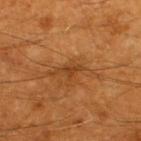  biopsy_status: not biopsied; imaged during a skin examination
  site: upper back
  patient:
    sex: male
    age_approx: 60
  lesion_size:
    long_diameter_mm_approx: 3.0
  lighting: cross-polarized
  automated_metrics:
    area_mm2_approx: 2.5
    eccentricity: 0.9
    shape_asymmetry: 0.45
    lesion_detection_confidence_0_100: 95
  image:
    source: total-body photography crop
    field_of_view_mm: 15